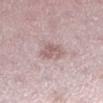Q: Is there a histopathology result?
A: catalogued during a skin exam; not biopsied
Q: Where on the body is the lesion?
A: the left lower leg
Q: What kind of image is this?
A: ~15 mm tile from a whole-body skin photo
Q: Who is the patient?
A: female, aged approximately 45